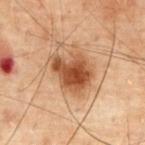Captured during whole-body skin photography for melanoma surveillance; the lesion was not biopsied.
A region of skin cropped from a whole-body photographic capture, roughly 15 mm wide.
Captured under cross-polarized illumination.
An algorithmic analysis of the crop reported an outline eccentricity of about 0.6 (0 = round, 1 = elongated) and a symmetry-axis asymmetry near 0.3. It also reported about 12 CIELAB-L* units darker than the surrounding skin and a normalized border contrast of about 10.5. The software also gave a border-irregularity index near 3.5/10 and a color-variation rating of about 4/10. And it measured a detector confidence of about 100 out of 100 that the crop contains a lesion.
A male patient aged around 70.
The lesion is on the chest.